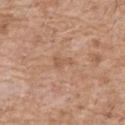  biopsy_status: not biopsied; imaged during a skin examination
  patient:
    sex: male
    age_approx: 60
  site: chest
  lesion_size:
    long_diameter_mm_approx: 2.5
  image:
    source: total-body photography crop
    field_of_view_mm: 15
  lighting: white-light